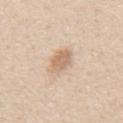No biopsy was performed on this lesion — it was imaged during a full skin examination and was not determined to be concerning. The lesion's longest dimension is about 3.5 mm. Captured under white-light illumination. Located on the back. This image is a 15 mm lesion crop taken from a total-body photograph. A male subject, in their 60s.